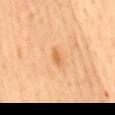notes: total-body-photography surveillance lesion; no biopsy
lesion diameter: about 2 mm
patient: male, about 60 years old
body site: the mid back
lighting: cross-polarized
image source: ~15 mm tile from a whole-body skin photo